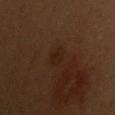No biopsy was performed on this lesion — it was imaged during a full skin examination and was not determined to be concerning.
Automated image analysis of the tile measured a lesion–skin lightness drop of about 4 and a normalized lesion–skin contrast near 5.5. And it measured a within-lesion color-variation index near 1/10 and peripheral color asymmetry of about 0.5.
A lesion tile, about 15 mm wide, cut from a 3D total-body photograph.
Imaged with cross-polarized lighting.
The subject is a female aged around 50.
On the left upper arm.
Longest diameter approximately 2.5 mm.